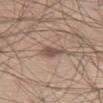| feature | finding |
|---|---|
| subject | male, in their 60s |
| lesion diameter | ≈3.5 mm |
| automated lesion analysis | an eccentricity of roughly 0.85 and two-axis asymmetry of about 0.3; border irregularity of about 3.5 on a 0–10 scale, a within-lesion color-variation index near 2/10, and peripheral color asymmetry of about 0.5; a nevus-likeness score of about 25/100 and lesion-presence confidence of about 100/100 |
| acquisition | ~15 mm tile from a whole-body skin photo |
| location | the left thigh |